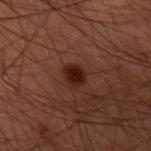notes: no biopsy performed (imaged during a skin exam)
lighting: cross-polarized
lesion size: ~3 mm (longest diameter)
location: the right forearm
patient: male, roughly 60 years of age
TBP lesion metrics: a lesion area of about 5 mm², an eccentricity of roughly 0.65, and a symmetry-axis asymmetry near 0.15; a normalized border contrast of about 11; border irregularity of about 1.5 on a 0–10 scale, a within-lesion color-variation index near 2.5/10, and radial color variation of about 0.5
imaging modality: ~15 mm crop, total-body skin-cancer survey